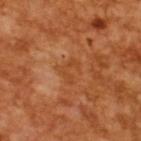Part of a total-body skin-imaging series; this lesion was reviewed on a skin check and was not flagged for biopsy. A male patient, in their mid-60s. The total-body-photography lesion software estimated border irregularity of about 6 on a 0–10 scale and radial color variation of about 0. A close-up tile cropped from a whole-body skin photograph, about 15 mm across.